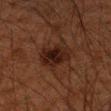Findings:
– notes: total-body-photography surveillance lesion; no biopsy
– subject: male, aged approximately 60
– image source: ~15 mm tile from a whole-body skin photo
– anatomic site: the right upper arm